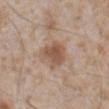Q: Was this lesion biopsied?
A: catalogued during a skin exam; not biopsied
Q: How was this image acquired?
A: ~15 mm crop, total-body skin-cancer survey
Q: Where on the body is the lesion?
A: the abdomen
Q: Patient demographics?
A: male, aged around 65
Q: Illumination type?
A: white-light illumination